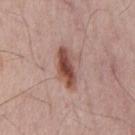workup: no biopsy performed (imaged during a skin exam) | body site: the chest | image: 15 mm crop, total-body photography | image-analysis metrics: a shape eccentricity near 0.85 and a shape-asymmetry score of about 0.2 (0 = symmetric); an average lesion color of about L≈50 a*≈22 b*≈25 (CIELAB), roughly 13 lightness units darker than nearby skin, and a lesion-to-skin contrast of about 9.5 (normalized; higher = more distinct); border irregularity of about 3 on a 0–10 scale and a color-variation rating of about 6.5/10; a classifier nevus-likeness of about 95/100 and lesion-presence confidence of about 100/100 | lesion diameter: about 5 mm | patient: male, aged 63–67 | tile lighting: white-light illumination.